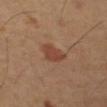<record>
  <lighting>cross-polarized</lighting>
  <automated_metrics>
    <cielab_L>40</cielab_L>
    <cielab_a>21</cielab_a>
    <cielab_b>28</cielab_b>
    <vs_skin_darker_L>8.0</vs_skin_darker_L>
    <vs_skin_contrast_norm>7.0</vs_skin_contrast_norm>
    <nevus_likeness_0_100>85</nevus_likeness_0_100>
    <lesion_detection_confidence_0_100>100</lesion_detection_confidence_0_100>
  </automated_metrics>
  <patient>
    <sex>male</sex>
    <age_approx>65</age_approx>
  </patient>
  <image>
    <source>total-body photography crop</source>
    <field_of_view_mm>15</field_of_view_mm>
  </image>
  <site>left upper arm</site>
</record>